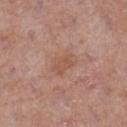Q: What are the patient's age and sex?
A: female, about 75 years old
Q: How was the tile lit?
A: white-light illumination
Q: What kind of image is this?
A: ~15 mm tile from a whole-body skin photo
Q: Lesion location?
A: the left lower leg